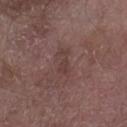workup = catalogued during a skin exam; not biopsied | image source = ~15 mm crop, total-body skin-cancer survey | patient = male, aged 63 to 67 | anatomic site = the right forearm.